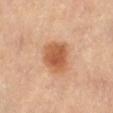| field | value |
|---|---|
| biopsy status | imaged on a skin check; not biopsied |
| subject | female, aged 48–52 |
| anatomic site | the lower back |
| acquisition | total-body-photography crop, ~15 mm field of view |